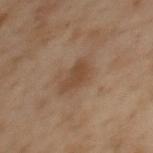Q: Is there a histopathology result?
A: imaged on a skin check; not biopsied
Q: What are the patient's age and sex?
A: female, aged 53 to 57
Q: What kind of image is this?
A: 15 mm crop, total-body photography
Q: How large is the lesion?
A: ≈3 mm
Q: What is the anatomic site?
A: the upper back